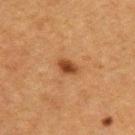| field | value |
|---|---|
| notes | total-body-photography surveillance lesion; no biopsy |
| anatomic site | the upper back |
| subject | male, aged approximately 50 |
| lighting | cross-polarized |
| lesion size | ~2.5 mm (longest diameter) |
| imaging modality | total-body-photography crop, ~15 mm field of view |